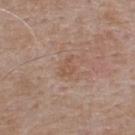{"biopsy_status": "not biopsied; imaged during a skin examination", "lighting": "white-light", "image": {"source": "total-body photography crop", "field_of_view_mm": 15}, "lesion_size": {"long_diameter_mm_approx": 2.5}, "automated_metrics": {"area_mm2_approx": 3.0, "eccentricity": 0.8, "border_irregularity_0_10": 5.5, "peripheral_color_asymmetry": 0.5, "nevus_likeness_0_100": 0}, "patient": {"sex": "male", "age_approx": 55}, "site": "upper back"}